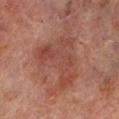Q: Was a biopsy performed?
A: total-body-photography surveillance lesion; no biopsy
Q: Who is the patient?
A: male, aged around 70
Q: What is the imaging modality?
A: 15 mm crop, total-body photography
Q: Lesion location?
A: the leg